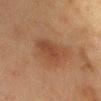Clinical impression:
This lesion was catalogued during total-body skin photography and was not selected for biopsy.
Background:
This image is a 15 mm lesion crop taken from a total-body photograph. A female subject, in their 50s. Located on the chest.